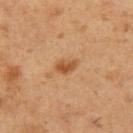Notes:
- biopsy status — no biopsy performed (imaged during a skin exam)
- image source — total-body-photography crop, ~15 mm field of view
- subject — male, approximately 55 years of age
- location — the left upper arm